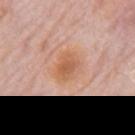{"biopsy_status": "not biopsied; imaged during a skin examination", "patient": {"sex": "male", "age_approx": 80}, "lesion_size": {"long_diameter_mm_approx": 4.0}, "automated_metrics": {"lesion_detection_confidence_0_100": 100}, "lighting": "white-light", "site": "mid back", "image": {"source": "total-body photography crop", "field_of_view_mm": 15}}